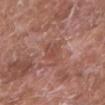{
  "image": {
    "source": "total-body photography crop",
    "field_of_view_mm": 15
  },
  "lesion_size": {
    "long_diameter_mm_approx": 4.5
  },
  "site": "arm",
  "patient": {
    "sex": "male",
    "age_approx": 80
  }
}